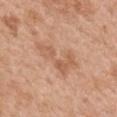Imaged during a routine full-body skin examination; the lesion was not biopsied and no histopathology is available. A female patient, roughly 40 years of age. Measured at roughly 6 mm in maximum diameter. This is a white-light tile. On the mid back. The total-body-photography lesion software estimated a border-irregularity index near 8.5/10, a color-variation rating of about 2.5/10, and a peripheral color-asymmetry measure near 0.5. A 15 mm close-up extracted from a 3D total-body photography capture.